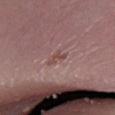Background:
The lesion is located on the leg. A 15 mm close-up extracted from a 3D total-body photography capture. A male subject, about 70 years old.